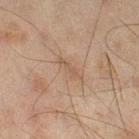Assessment:
This lesion was catalogued during total-body skin photography and was not selected for biopsy.
Clinical summary:
This is a cross-polarized tile. Automated image analysis of the tile measured a mean CIELAB color near L≈44 a*≈13 b*≈25, about 5 CIELAB-L* units darker than the surrounding skin, and a lesion-to-skin contrast of about 4.5 (normalized; higher = more distinct). The software also gave a border-irregularity index near 4/10, a within-lesion color-variation index near 0/10, and radial color variation of about 0. A close-up tile cropped from a whole-body skin photograph, about 15 mm across. On the right thigh. A male subject, in their mid- to late 40s. Measured at roughly 3.5 mm in maximum diameter.